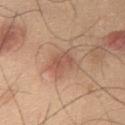Findings:
– workup: no biopsy performed (imaged during a skin exam)
– location: the chest
– patient: male, aged 43 to 47
– image: ~15 mm tile from a whole-body skin photo